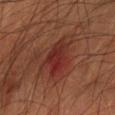Impression: This lesion was catalogued during total-body skin photography and was not selected for biopsy. Background: A 15 mm close-up tile from a total-body photography series done for melanoma screening. Located on the right forearm. The tile uses cross-polarized illumination. An algorithmic analysis of the crop reported an area of roughly 11 mm², an eccentricity of roughly 0.8, and a symmetry-axis asymmetry near 0.25. The software also gave an average lesion color of about L≈28 a*≈26 b*≈24 (CIELAB), a lesion–skin lightness drop of about 7, and a normalized lesion–skin contrast near 7.5. About 5 mm across. The subject is a male about 65 years old.